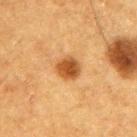{
  "patient": {
    "sex": "male",
    "age_approx": 75
  },
  "site": "right upper arm",
  "image": {
    "source": "total-body photography crop",
    "field_of_view_mm": 15
  },
  "automated_metrics": {
    "area_mm2_approx": 6.0,
    "eccentricity": 0.55,
    "shape_asymmetry": 0.2,
    "cielab_L": 43,
    "cielab_a": 21,
    "cielab_b": 37,
    "vs_skin_contrast_norm": 10.0,
    "nevus_likeness_0_100": 100,
    "lesion_detection_confidence_0_100": 100
  },
  "lesion_size": {
    "long_diameter_mm_approx": 3.0
  }
}